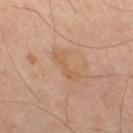Assessment:
The lesion was tiled from a total-body skin photograph and was not biopsied.
Clinical summary:
A roughly 15 mm field-of-view crop from a total-body skin photograph. A male subject in their mid- to late 60s. From the leg. The recorded lesion diameter is about 4.5 mm.